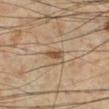Recorded during total-body skin imaging; not selected for excision or biopsy. Imaged with cross-polarized lighting. The subject is a male aged around 55. A 15 mm close-up tile from a total-body photography series done for melanoma screening. The lesion-visualizer software estimated an average lesion color of about L≈51 a*≈17 b*≈33 (CIELAB), a lesion–skin lightness drop of about 11, and a normalized lesion–skin contrast near 8. And it measured border irregularity of about 3.5 on a 0–10 scale, a color-variation rating of about 0/10, and a peripheral color-asymmetry measure near 0. Located on the left lower leg.